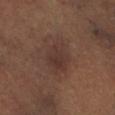Clinical impression:
Part of a total-body skin-imaging series; this lesion was reviewed on a skin check and was not flagged for biopsy.
Image and clinical context:
Measured at roughly 4.5 mm in maximum diameter. A female subject, about 65 years old. Located on the left lower leg. The tile uses cross-polarized illumination. A roughly 15 mm field-of-view crop from a total-body skin photograph.